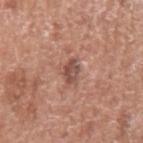Located on the arm. The recorded lesion diameter is about 3 mm. Captured under white-light illumination. A male subject, in their mid- to late 70s. A roughly 15 mm field-of-view crop from a total-body skin photograph.